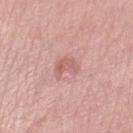Clinical impression:
Recorded during total-body skin imaging; not selected for excision or biopsy.
Acquisition and patient details:
The lesion's longest dimension is about 3 mm. The patient is a female aged 38 to 42. This image is a 15 mm lesion crop taken from a total-body photograph. On the left lower leg. This is a white-light tile. The lesion-visualizer software estimated a footprint of about 5 mm² and a symmetry-axis asymmetry near 0.4. The analysis additionally found a lesion color around L≈61 a*≈25 b*≈24 in CIELAB, about 8 CIELAB-L* units darker than the surrounding skin, and a lesion-to-skin contrast of about 5.5 (normalized; higher = more distinct). It also reported a border-irregularity index near 4/10, a color-variation rating of about 2.5/10, and a peripheral color-asymmetry measure near 1. The analysis additionally found a nevus-likeness score of about 0/100 and lesion-presence confidence of about 100/100.